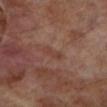workup = no biopsy performed (imaged during a skin exam) | patient = male, aged approximately 70 | imaging modality = ~15 mm tile from a whole-body skin photo | site = the left lower leg | illumination = cross-polarized illumination | lesion size = ≈2.5 mm | image-analysis metrics = a within-lesion color-variation index near 0/10 and radial color variation of about 0; an automated nevus-likeness rating near 0 out of 100 and lesion-presence confidence of about 100/100.